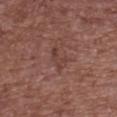{"biopsy_status": "not biopsied; imaged during a skin examination", "site": "upper back", "lesion_size": {"long_diameter_mm_approx": 3.0}, "lighting": "white-light", "automated_metrics": {"area_mm2_approx": 3.5, "eccentricity": 0.9, "shape_asymmetry": 0.5, "cielab_L": 40, "cielab_a": 21, "cielab_b": 23, "vs_skin_darker_L": 6.0, "vs_skin_contrast_norm": 5.0, "border_irregularity_0_10": 6.0, "color_variation_0_10": 0.0, "peripheral_color_asymmetry": 0.0, "nevus_likeness_0_100": 0, "lesion_detection_confidence_0_100": 100}, "image": {"source": "total-body photography crop", "field_of_view_mm": 15}, "patient": {"sex": "female", "age_approx": 75}}